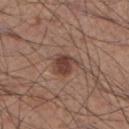Captured during whole-body skin photography for melanoma surveillance; the lesion was not biopsied. Captured under white-light illumination. The lesion is located on the left lower leg. The patient is a male in their mid-30s. About 3.5 mm across. A lesion tile, about 15 mm wide, cut from a 3D total-body photograph. An algorithmic analysis of the crop reported a lesion area of about 6 mm², an outline eccentricity of about 0.65 (0 = round, 1 = elongated), and a symmetry-axis asymmetry near 0.25. It also reported an automated nevus-likeness rating near 90 out of 100.